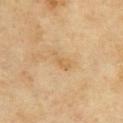| feature | finding |
|---|---|
| patient | female, aged 58 to 62 |
| diameter | about 3.5 mm |
| location | the upper back |
| imaging modality | ~15 mm tile from a whole-body skin photo |
| lighting | cross-polarized |